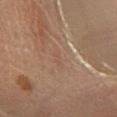This lesion was catalogued during total-body skin photography and was not selected for biopsy. A 15 mm close-up tile from a total-body photography series done for melanoma screening. The patient is a male in their 60s. From the right lower leg.